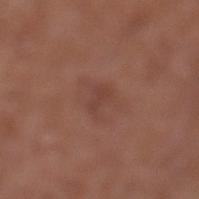workup: total-body-photography surveillance lesion; no biopsy | image source: ~15 mm tile from a whole-body skin photo | site: the left lower leg | diameter: ~2.5 mm (longest diameter) | automated metrics: an area of roughly 3.5 mm², an outline eccentricity of about 0.8 (0 = round, 1 = elongated), and two-axis asymmetry of about 0.25; roughly 5 lightness units darker than nearby skin; lesion-presence confidence of about 100/100 | illumination: white-light illumination | patient: female, aged 78–82.